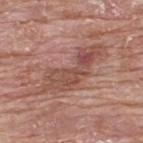Case summary:
• biopsy status · imaged on a skin check; not biopsied
• site · the upper back
• tile lighting · white-light illumination
• image source · ~15 mm crop, total-body skin-cancer survey
• patient · male, aged 63 to 67